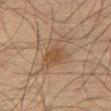Background:
A male patient aged approximately 65. Captured under cross-polarized illumination. About 3 mm across. A lesion tile, about 15 mm wide, cut from a 3D total-body photograph. Located on the right thigh.